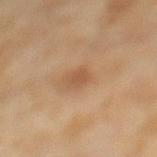| feature | finding |
|---|---|
| biopsy status | imaged on a skin check; not biopsied |
| anatomic site | the left lower leg |
| illumination | cross-polarized |
| lesion diameter | ≈2.5 mm |
| automated metrics | a footprint of about 3.5 mm², an eccentricity of roughly 0.75, and two-axis asymmetry of about 0.25; a classifier nevus-likeness of about 50/100 and a detector confidence of about 100 out of 100 that the crop contains a lesion |
| image source | ~15 mm tile from a whole-body skin photo |
| subject | female, aged 58 to 62 |